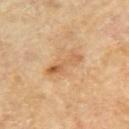Impression:
Captured during whole-body skin photography for melanoma surveillance; the lesion was not biopsied.
Acquisition and patient details:
The lesion's longest dimension is about 4 mm. The lesion is located on the left upper arm. The tile uses cross-polarized illumination. A male subject about 70 years old. A roughly 15 mm field-of-view crop from a total-body skin photograph.